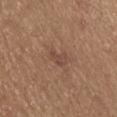Cropped from a whole-body photographic skin survey; the tile spans about 15 mm.
From the abdomen.
A male patient, in their mid- to late 60s.